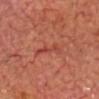| key | value |
|---|---|
| notes | imaged on a skin check; not biopsied |
| automated lesion analysis | an average lesion color of about L≈44 a*≈32 b*≈31 (CIELAB) and a lesion–skin lightness drop of about 6 |
| image | total-body-photography crop, ~15 mm field of view |
| site | the head or neck |
| diameter | ≈4 mm |
| illumination | cross-polarized illumination |
| subject | male, aged 58 to 62 |